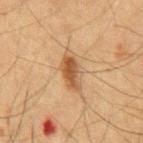biopsy_status: not biopsied; imaged during a skin examination
image:
  source: total-body photography crop
  field_of_view_mm: 15
patient:
  sex: male
  age_approx: 65
lighting: cross-polarized
automated_metrics:
  area_mm2_approx: 8.5
  eccentricity: 0.85
  border_irregularity_0_10: 2.5
  color_variation_0_10: 5.0
  nevus_likeness_0_100: 90
lesion_size:
  long_diameter_mm_approx: 5.0
site: chest